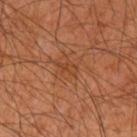Impression: This lesion was catalogued during total-body skin photography and was not selected for biopsy. Context: About 2.5 mm across. A male patient, approximately 60 years of age. This is a cross-polarized tile. A close-up tile cropped from a whole-body skin photograph, about 15 mm across. From the left upper arm.